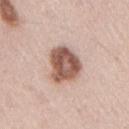Captured during whole-body skin photography for melanoma surveillance; the lesion was not biopsied. A 15 mm crop from a total-body photograph taken for skin-cancer surveillance. Measured at roughly 4.5 mm in maximum diameter. The lesion is on the right upper arm. Captured under white-light illumination. A male patient aged 43–47.